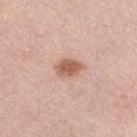workup: imaged on a skin check; not biopsied